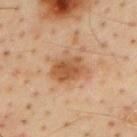The lesion was photographed on a routine skin check and not biopsied; there is no pathology result. A male subject about 55 years old. A region of skin cropped from a whole-body photographic capture, roughly 15 mm wide. Measured at roughly 4 mm in maximum diameter. The lesion is located on the mid back.